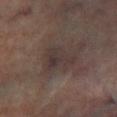The lesion was tiled from a total-body skin photograph and was not biopsied. Measured at roughly 4.5 mm in maximum diameter. Located on the left lower leg. This is a cross-polarized tile. A male subject, in their mid- to late 60s. Automated tile analysis of the lesion measured a lesion color around L≈31 a*≈12 b*≈15 in CIELAB, a lesion–skin lightness drop of about 6, and a lesion-to-skin contrast of about 6.5 (normalized; higher = more distinct). The software also gave a border-irregularity index near 4.5/10 and a within-lesion color-variation index near 4/10. The analysis additionally found an automated nevus-likeness rating near 0 out of 100 and lesion-presence confidence of about 55/100. A close-up tile cropped from a whole-body skin photograph, about 15 mm across.